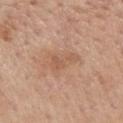Q: Is there a histopathology result?
A: no biopsy performed (imaged during a skin exam)
Q: What kind of image is this?
A: total-body-photography crop, ~15 mm field of view
Q: What is the anatomic site?
A: the mid back
Q: What did automated image analysis measure?
A: a lesion area of about 5.5 mm², a shape eccentricity near 0.9, and two-axis asymmetry of about 0.6; a mean CIELAB color near L≈57 a*≈21 b*≈32, a lesion–skin lightness drop of about 7, and a lesion-to-skin contrast of about 5 (normalized; higher = more distinct); a border-irregularity index near 6.5/10, a color-variation rating of about 1.5/10, and radial color variation of about 0.5
Q: Illumination type?
A: white-light
Q: Lesion size?
A: about 4 mm
Q: Patient demographics?
A: female, aged 63–67